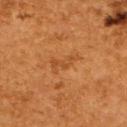Notes:
– biopsy status — no biopsy performed (imaged during a skin exam)
– acquisition — ~15 mm tile from a whole-body skin photo
– illumination — cross-polarized
– patient — female, aged 48–52
– body site — the upper back